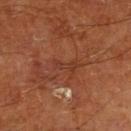Q: Is there a histopathology result?
A: total-body-photography surveillance lesion; no biopsy
Q: Lesion location?
A: the left lower leg
Q: What kind of image is this?
A: ~15 mm crop, total-body skin-cancer survey
Q: How was the tile lit?
A: cross-polarized
Q: Lesion size?
A: ≈3 mm
Q: What are the patient's age and sex?
A: male, about 65 years old